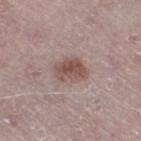Findings:
• biopsy status: imaged on a skin check; not biopsied
• imaging modality: ~15 mm tile from a whole-body skin photo
• size: ≈3.5 mm
• anatomic site: the right thigh
• patient: male, aged 73–77